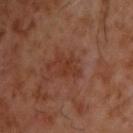Clinical impression: No biopsy was performed on this lesion — it was imaged during a full skin examination and was not determined to be concerning. Image and clinical context: Cropped from a whole-body photographic skin survey; the tile spans about 15 mm. The subject is a male approximately 60 years of age. Captured under cross-polarized illumination. From the upper back. The lesion's longest dimension is about 3.5 mm.